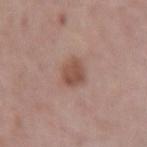workup: catalogued during a skin exam; not biopsied
anatomic site: the right forearm
subject: female, in their 40s
acquisition: ~15 mm crop, total-body skin-cancer survey
lighting: white-light
size: ≈3 mm